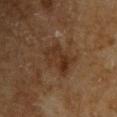Findings:
* automated metrics — an area of roughly 10 mm², a shape eccentricity near 0.8, and two-axis asymmetry of about 0.3; a lesion color around L≈28 a*≈16 b*≈26 in CIELAB and roughly 7 lightness units darker than nearby skin; an automated nevus-likeness rating near 5 out of 100 and lesion-presence confidence of about 100/100
* lesion size — about 5 mm
* illumination — cross-polarized illumination
* imaging modality — 15 mm crop, total-body photography
* anatomic site — the upper back
* patient — male, aged 63–67